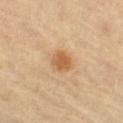biopsy_status: not biopsied; imaged during a skin examination
site: right thigh
lighting: cross-polarized
automated_metrics:
  area_mm2_approx: 6.0
  eccentricity: 0.65
  shape_asymmetry: 0.25
  cielab_L: 61
  cielab_a: 20
  cielab_b: 40
  vs_skin_darker_L: 11.0
  vs_skin_contrast_norm: 7.5
  nevus_likeness_0_100: 90
  lesion_detection_confidence_0_100: 100
patient:
  sex: female
  age_approx: 70
image:
  source: total-body photography crop
  field_of_view_mm: 15